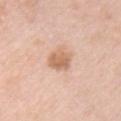Clinical impression: This lesion was catalogued during total-body skin photography and was not selected for biopsy. Clinical summary: Imaged with white-light lighting. The subject is a female aged approximately 40. A roughly 15 mm field-of-view crop from a total-body skin photograph. Approximately 3 mm at its widest. Automated image analysis of the tile measured an eccentricity of roughly 0.45 and a symmetry-axis asymmetry near 0.2. The analysis additionally found an average lesion color of about L≈65 a*≈20 b*≈33 (CIELAB), roughly 11 lightness units darker than nearby skin, and a normalized lesion–skin contrast near 7.5. And it measured a border-irregularity rating of about 1.5/10, a within-lesion color-variation index near 3/10, and peripheral color asymmetry of about 1. On the left arm.